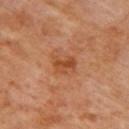Q: Was a biopsy performed?
A: total-body-photography surveillance lesion; no biopsy
Q: What lighting was used for the tile?
A: cross-polarized
Q: Where on the body is the lesion?
A: the upper back
Q: Patient demographics?
A: female, approximately 65 years of age
Q: What is the imaging modality?
A: total-body-photography crop, ~15 mm field of view